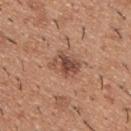Recorded during total-body skin imaging; not selected for excision or biopsy. From the upper back. A male patient aged around 40. A lesion tile, about 15 mm wide, cut from a 3D total-body photograph.